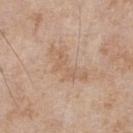Clinical impression: Part of a total-body skin-imaging series; this lesion was reviewed on a skin check and was not flagged for biopsy. Image and clinical context: The lesion-visualizer software estimated a border-irregularity index near 7.5/10, a within-lesion color-variation index near 2/10, and peripheral color asymmetry of about 0.5. It also reported a classifier nevus-likeness of about 0/100. A region of skin cropped from a whole-body photographic capture, roughly 15 mm wide. Longest diameter approximately 5.5 mm. From the front of the torso. A male subject aged 63 to 67.